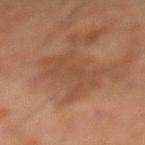biopsy_status: not biopsied; imaged during a skin examination
patient:
  sex: male
  age_approx: 60
automated_metrics:
  area_mm2_approx: 13.0
  eccentricity: 0.9
  shape_asymmetry: 0.4
  cielab_L: 44
  cielab_a: 19
  cielab_b: 31
  vs_skin_darker_L: 6.0
  vs_skin_contrast_norm: 5.0
  border_irregularity_0_10: 8.0
  color_variation_0_10: 1.5
  peripheral_color_asymmetry: 0.5
site: left lower leg
image:
  source: total-body photography crop
  field_of_view_mm: 15